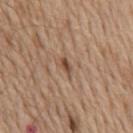Impression: This lesion was catalogued during total-body skin photography and was not selected for biopsy. Acquisition and patient details: A region of skin cropped from a whole-body photographic capture, roughly 15 mm wide. The subject is a male roughly 70 years of age. Located on the chest.